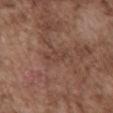Recorded during total-body skin imaging; not selected for excision or biopsy. From the chest. This is a white-light tile. A male patient, aged 73 to 77. About 3.5 mm across. This image is a 15 mm lesion crop taken from a total-body photograph.